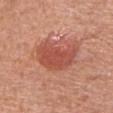Findings:
- notes: no biopsy performed (imaged during a skin exam)
- image source: total-body-photography crop, ~15 mm field of view
- tile lighting: white-light illumination
- anatomic site: the left upper arm
- patient: male, roughly 55 years of age
- size: ≈5.5 mm
- automated metrics: a shape eccentricity near 0.8 and a shape-asymmetry score of about 0.35 (0 = symmetric); about 11 CIELAB-L* units darker than the surrounding skin and a normalized border contrast of about 7.5; a border-irregularity rating of about 4/10, internal color variation of about 3 on a 0–10 scale, and peripheral color asymmetry of about 1